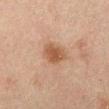This lesion was catalogued during total-body skin photography and was not selected for biopsy.
The lesion's longest dimension is about 3 mm.
The lesion-visualizer software estimated a mean CIELAB color near L≈41 a*≈17 b*≈27, roughly 9 lightness units darker than nearby skin, and a lesion-to-skin contrast of about 8 (normalized; higher = more distinct). And it measured a within-lesion color-variation index near 2/10 and peripheral color asymmetry of about 0.5.
A 15 mm crop from a total-body photograph taken for skin-cancer surveillance.
The tile uses cross-polarized illumination.
The patient is a male aged 48–52.
Located on the abdomen.